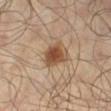Acquisition and patient details:
A region of skin cropped from a whole-body photographic capture, roughly 15 mm wide. The tile uses cross-polarized illumination. The patient is a male approximately 65 years of age. On the leg.